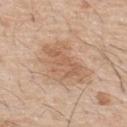Q: Patient demographics?
A: male, aged 53 to 57
Q: How large is the lesion?
A: ~5.5 mm (longest diameter)
Q: Lesion location?
A: the upper back
Q: Automated lesion metrics?
A: a footprint of about 14 mm² and an eccentricity of roughly 0.75; an average lesion color of about L≈60 a*≈19 b*≈32 (CIELAB) and a normalized border contrast of about 5.5; a color-variation rating of about 3/10 and peripheral color asymmetry of about 1; an automated nevus-likeness rating near 0 out of 100 and lesion-presence confidence of about 100/100
Q: What is the imaging modality?
A: total-body-photography crop, ~15 mm field of view